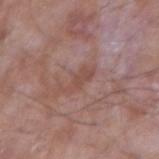Impression:
The lesion was photographed on a routine skin check and not biopsied; there is no pathology result.
Image and clinical context:
A male subject roughly 65 years of age. A 15 mm crop from a total-body photograph taken for skin-cancer surveillance. About 4 mm across. The lesion is located on the arm.